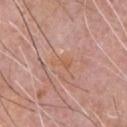  biopsy_status: not biopsied; imaged during a skin examination